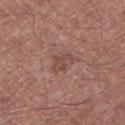Q: Was this lesion biopsied?
A: imaged on a skin check; not biopsied
Q: What did automated image analysis measure?
A: a border-irregularity index near 3/10, a within-lesion color-variation index near 2/10, and a peripheral color-asymmetry measure near 0.5; a nevus-likeness score of about 0/100 and a detector confidence of about 100 out of 100 that the crop contains a lesion
Q: How was the tile lit?
A: white-light illumination
Q: Where on the body is the lesion?
A: the leg
Q: What kind of image is this?
A: ~15 mm crop, total-body skin-cancer survey
Q: What are the patient's age and sex?
A: male, roughly 55 years of age
Q: What is the lesion's diameter?
A: ~3 mm (longest diameter)